The lesion is on the head or neck, the subject is a male aged 68 to 72, a 15 mm close-up tile from a total-body photography series done for melanoma screening — 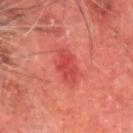TBP lesion metrics: a lesion area of about 6.5 mm², an eccentricity of roughly 0.75, and a symmetry-axis asymmetry near 0.3; a lesion–skin lightness drop of about 8 and a normalized border contrast of about 6; a classifier nevus-likeness of about 0/100 and lesion-presence confidence of about 100/100
illumination: cross-polarized
size: about 3.5 mm
diagnosis: a nodular basal cell carcinoma (malignant)